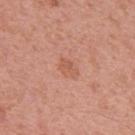Case summary:
– biopsy status — catalogued during a skin exam; not biopsied
– diameter — about 2.5 mm
– TBP lesion metrics — a lesion color around L≈57 a*≈26 b*≈32 in CIELAB, a lesion–skin lightness drop of about 7, and a lesion-to-skin contrast of about 5 (normalized; higher = more distinct)
– illumination — white-light illumination
– anatomic site — the back
– subject — male, aged 53–57
– acquisition — ~15 mm tile from a whole-body skin photo